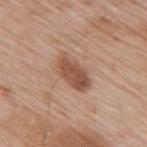Q: Is there a histopathology result?
A: total-body-photography surveillance lesion; no biopsy
Q: Lesion location?
A: the upper back
Q: How was the tile lit?
A: white-light illumination
Q: What kind of image is this?
A: ~15 mm crop, total-body skin-cancer survey
Q: What is the lesion's diameter?
A: ≈4.5 mm
Q: Automated lesion metrics?
A: an area of roughly 8.5 mm² and a shape eccentricity near 0.85; an average lesion color of about L≈51 a*≈22 b*≈30 (CIELAB), roughly 12 lightness units darker than nearby skin, and a normalized lesion–skin contrast near 8.5; a color-variation rating of about 4/10 and a peripheral color-asymmetry measure near 1.5; an automated nevus-likeness rating near 70 out of 100 and lesion-presence confidence of about 100/100
Q: What are the patient's age and sex?
A: male, about 80 years old